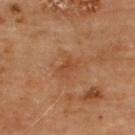Q: Was a biopsy performed?
A: imaged on a skin check; not biopsied
Q: What is the anatomic site?
A: the upper back
Q: What kind of image is this?
A: ~15 mm crop, total-body skin-cancer survey
Q: Lesion size?
A: ~2.5 mm (longest diameter)
Q: Who is the patient?
A: male, aged 63–67